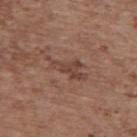Measured at roughly 5 mm in maximum diameter. The lesion is on the upper back. An algorithmic analysis of the crop reported a lesion area of about 7 mm², a shape eccentricity near 0.9, and a symmetry-axis asymmetry near 0.45. The software also gave a lesion–skin lightness drop of about 8. The software also gave a border-irregularity rating of about 6.5/10, internal color variation of about 2 on a 0–10 scale, and radial color variation of about 0.5. Captured under white-light illumination. The patient is a female in their mid- to late 60s. A roughly 15 mm field-of-view crop from a total-body skin photograph.